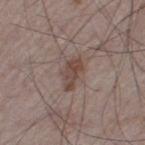Part of a total-body skin-imaging series; this lesion was reviewed on a skin check and was not flagged for biopsy.
Captured under white-light illumination.
From the left thigh.
A lesion tile, about 15 mm wide, cut from a 3D total-body photograph.
The lesion-visualizer software estimated a lesion–skin lightness drop of about 9 and a normalized border contrast of about 7.5. It also reported a detector confidence of about 100 out of 100 that the crop contains a lesion.
A male patient, about 75 years old.
Approximately 4 mm at its widest.